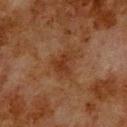Q: Is there a histopathology result?
A: no biopsy performed (imaged during a skin exam)
Q: What is the anatomic site?
A: the upper back
Q: What did automated image analysis measure?
A: an average lesion color of about L≈27 a*≈19 b*≈27 (CIELAB), roughly 6 lightness units darker than nearby skin, and a lesion-to-skin contrast of about 6.5 (normalized; higher = more distinct); a border-irregularity rating of about 4.5/10, a within-lesion color-variation index near 1.5/10, and radial color variation of about 0.5
Q: How was this image acquired?
A: total-body-photography crop, ~15 mm field of view
Q: Lesion size?
A: ~3 mm (longest diameter)
Q: What are the patient's age and sex?
A: male, about 80 years old
Q: What lighting was used for the tile?
A: cross-polarized illumination